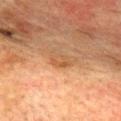Clinical impression: This lesion was catalogued during total-body skin photography and was not selected for biopsy. Context: Cropped from a whole-body photographic skin survey; the tile spans about 15 mm. From the upper back. A male subject aged 73–77.